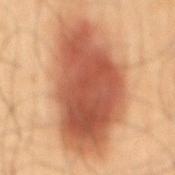Assessment: Captured during whole-body skin photography for melanoma surveillance; the lesion was not biopsied. Acquisition and patient details: The total-body-photography lesion software estimated a footprint of about 65 mm² and an outline eccentricity of about 0.85 (0 = round, 1 = elongated). The software also gave a lesion color around L≈54 a*≈26 b*≈34 in CIELAB, about 17 CIELAB-L* units darker than the surrounding skin, and a lesion-to-skin contrast of about 11 (normalized; higher = more distinct). The analysis additionally found a border-irregularity rating of about 3/10 and internal color variation of about 7 on a 0–10 scale. This image is a 15 mm lesion crop taken from a total-body photograph. Measured at roughly 12 mm in maximum diameter. The lesion is located on the back. This is a cross-polarized tile. A male subject, aged 58–62.